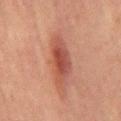Impression:
The lesion was photographed on a routine skin check and not biopsied; there is no pathology result.
Clinical summary:
A male subject, about 65 years old. Cropped from a whole-body photographic skin survey; the tile spans about 15 mm. Approximately 5.5 mm at its widest. The total-body-photography lesion software estimated roughly 9 lightness units darker than nearby skin and a normalized lesion–skin contrast near 7.5. The software also gave a border-irregularity index near 3.5/10, a within-lesion color-variation index near 5/10, and radial color variation of about 1.5. It also reported a classifier nevus-likeness of about 80/100. The lesion is located on the front of the torso.